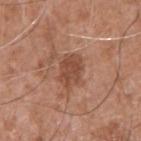Part of a total-body skin-imaging series; this lesion was reviewed on a skin check and was not flagged for biopsy.
Automated tile analysis of the lesion measured a symmetry-axis asymmetry near 0.25. The software also gave an automated nevus-likeness rating near 20 out of 100 and a lesion-detection confidence of about 100/100.
Approximately 4 mm at its widest.
A male patient, aged around 75.
On the right upper arm.
A 15 mm close-up extracted from a 3D total-body photography capture.
Imaged with white-light lighting.